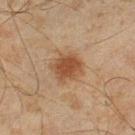No biopsy was performed on this lesion — it was imaged during a full skin examination and was not determined to be concerning.